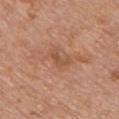  biopsy_status: not biopsied; imaged during a skin examination
  site: front of the torso
  lighting: white-light
  automated_metrics:
    area_mm2_approx: 3.5
    eccentricity: 0.8
    shape_asymmetry: 0.55
    cielab_L: 52
    cielab_a: 22
    cielab_b: 32
    vs_skin_darker_L: 7.0
    vs_skin_contrast_norm: 5.0
    border_irregularity_0_10: 6.0
    peripheral_color_asymmetry: 0.0
    nevus_likeness_0_100: 0
    lesion_detection_confidence_0_100: 100
  patient:
    sex: male
    age_approx: 60
  image:
    source: total-body photography crop
    field_of_view_mm: 15